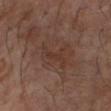image: 15 mm crop, total-body photography | illumination: cross-polarized | site: the left forearm | patient: male, in their mid-60s.